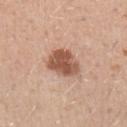Imaged during a routine full-body skin examination; the lesion was not biopsied and no histopathology is available. This image is a 15 mm lesion crop taken from a total-body photograph. The subject is a male approximately 30 years of age. On the left upper arm.